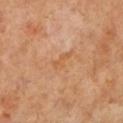Clinical impression:
The lesion was tiled from a total-body skin photograph and was not biopsied.
Acquisition and patient details:
A 15 mm close-up tile from a total-body photography series done for melanoma screening. Imaged with cross-polarized lighting. From the left lower leg. A female patient roughly 55 years of age. Automated tile analysis of the lesion measured a footprint of about 3.5 mm², an eccentricity of roughly 0.9, and a symmetry-axis asymmetry near 0.45. It also reported border irregularity of about 4.5 on a 0–10 scale, a within-lesion color-variation index near 0/10, and a peripheral color-asymmetry measure near 0. Longest diameter approximately 3 mm.